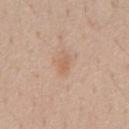Part of a total-body skin-imaging series; this lesion was reviewed on a skin check and was not flagged for biopsy. The total-body-photography lesion software estimated a footprint of about 2.5 mm², a shape eccentricity near 0.9, and a shape-asymmetry score of about 0.35 (0 = symmetric). The software also gave a lesion color around L≈61 a*≈19 b*≈32 in CIELAB and a lesion-to-skin contrast of about 5.5 (normalized; higher = more distinct). It also reported a classifier nevus-likeness of about 5/100 and lesion-presence confidence of about 100/100. On the abdomen. The patient is a male aged approximately 55. Approximately 3 mm at its widest. This is a white-light tile. A roughly 15 mm field-of-view crop from a total-body skin photograph.